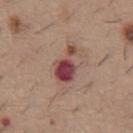Assessment: Imaged during a routine full-body skin examination; the lesion was not biopsied and no histopathology is available. Clinical summary: A 15 mm close-up extracted from a 3D total-body photography capture. A male patient, aged 58 to 62. Measured at roughly 4 mm in maximum diameter. The lesion is on the abdomen.